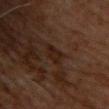No biopsy was performed on this lesion — it was imaged during a full skin examination and was not determined to be concerning.
The lesion is located on the upper back.
Approximately 2.5 mm at its widest.
A 15 mm close-up tile from a total-body photography series done for melanoma screening.
A male patient, aged around 60.
Captured under cross-polarized illumination.